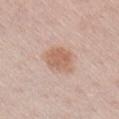Recorded during total-body skin imaging; not selected for excision or biopsy.
Located on the arm.
A lesion tile, about 15 mm wide, cut from a 3D total-body photograph.
A male subject about 60 years old.